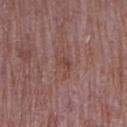| feature | finding |
|---|---|
| follow-up | imaged on a skin check; not biopsied |
| acquisition | 15 mm crop, total-body photography |
| subject | female, approximately 70 years of age |
| tile lighting | white-light |
| body site | the right upper arm |
| lesion diameter | ≈3.5 mm |